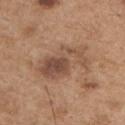Recorded during total-body skin imaging; not selected for excision or biopsy. The tile uses white-light illumination. A male patient aged 63–67. Automated image analysis of the tile measured an area of roughly 18 mm², an eccentricity of roughly 0.85, and a shape-asymmetry score of about 0.35 (0 = symmetric). A close-up tile cropped from a whole-body skin photograph, about 15 mm across. Located on the chest.